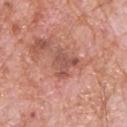{"biopsy_status": "not biopsied; imaged during a skin examination", "lighting": "white-light", "site": "upper back", "patient": {"sex": "male", "age_approx": 80}, "image": {"source": "total-body photography crop", "field_of_view_mm": 15}, "automated_metrics": {"eccentricity": 0.6, "shape_asymmetry": 0.45, "vs_skin_contrast_norm": 6.5}}